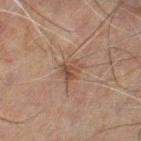Q: Was this lesion biopsied?
A: total-body-photography surveillance lesion; no biopsy
Q: Where on the body is the lesion?
A: the left thigh
Q: Illumination type?
A: cross-polarized illumination
Q: Patient demographics?
A: male, about 60 years old
Q: What kind of image is this?
A: ~15 mm crop, total-body skin-cancer survey